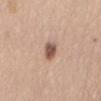Automated tile analysis of the lesion measured a footprint of about 4.5 mm² and two-axis asymmetry of about 0.2. The software also gave a border-irregularity index near 2/10. The software also gave a nevus-likeness score of about 75/100.
The lesion's longest dimension is about 3 mm.
The tile uses white-light illumination.
A female patient, approximately 50 years of age.
The lesion is located on the abdomen.
Cropped from a total-body skin-imaging series; the visible field is about 15 mm.